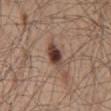<case>
  <biopsy_status>not biopsied; imaged during a skin examination</biopsy_status>
  <image>
    <source>total-body photography crop</source>
    <field_of_view_mm>15</field_of_view_mm>
  </image>
  <lesion_size>
    <long_diameter_mm_approx>3.5</long_diameter_mm_approx>
  </lesion_size>
  <patient>
    <sex>male</sex>
    <age_approx>50</age_approx>
  </patient>
  <lighting>white-light</lighting>
  <site>back</site>
</case>